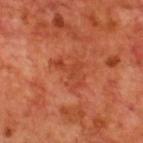Assessment:
No biopsy was performed on this lesion — it was imaged during a full skin examination and was not determined to be concerning.
Context:
The lesion is located on the upper back. Captured under cross-polarized illumination. Cropped from a total-body skin-imaging series; the visible field is about 15 mm. A male subject, approximately 70 years of age.